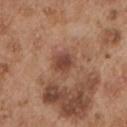Clinical impression:
The lesion was photographed on a routine skin check and not biopsied; there is no pathology result.
Image and clinical context:
The lesion is on the left upper arm. A 15 mm close-up extracted from a 3D total-body photography capture. A male patient roughly 55 years of age.